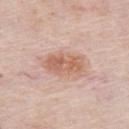illumination = white-light illumination | body site = the front of the torso | image = ~15 mm tile from a whole-body skin photo | image-analysis metrics = a border-irregularity index near 2/10 and a peripheral color-asymmetry measure near 1.5 | patient = male, roughly 80 years of age | diameter = ~5.5 mm (longest diameter).